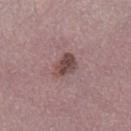<lesion>
  <automated_metrics>
    <cielab_L>46</cielab_L>
    <cielab_a>20</cielab_a>
    <cielab_b>18</cielab_b>
    <vs_skin_contrast_norm>8.5</vs_skin_contrast_norm>
    <border_irregularity_0_10>2.5</border_irregularity_0_10>
    <color_variation_0_10>4.0</color_variation_0_10>
    <peripheral_color_asymmetry>1.0</peripheral_color_asymmetry>
  </automated_metrics>
  <patient>
    <sex>female</sex>
    <age_approx>50</age_approx>
  </patient>
  <site>right lower leg</site>
  <image>
    <source>total-body photography crop</source>
    <field_of_view_mm>15</field_of_view_mm>
  </image>
  <lesion_size>
    <long_diameter_mm_approx>3.0</long_diameter_mm_approx>
  </lesion_size>
</lesion>